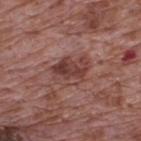No biopsy was performed on this lesion — it was imaged during a full skin examination and was not determined to be concerning. The lesion is located on the upper back. A roughly 15 mm field-of-view crop from a total-body skin photograph. This is a white-light tile. Automated image analysis of the tile measured a classifier nevus-likeness of about 30/100 and a lesion-detection confidence of about 100/100. The lesion's longest dimension is about 4.5 mm. A male patient, aged 68 to 72.